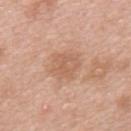follow-up — imaged on a skin check; not biopsied | image source — 15 mm crop, total-body photography | site — the back | automated lesion analysis — a footprint of about 8.5 mm², a shape eccentricity near 0.45, and a shape-asymmetry score of about 0.25 (0 = symmetric); a border-irregularity index near 2.5/10, a within-lesion color-variation index near 3/10, and peripheral color asymmetry of about 1 | patient — male, approximately 50 years of age | lesion diameter — ≈3.5 mm.